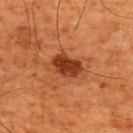Recorded during total-body skin imaging; not selected for excision or biopsy.
From the back.
Imaged with cross-polarized lighting.
The recorded lesion diameter is about 4.5 mm.
A region of skin cropped from a whole-body photographic capture, roughly 15 mm wide.
A male patient about 65 years old.